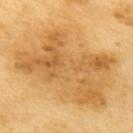Notes:
• follow-up — catalogued during a skin exam; not biopsied
• lighting — cross-polarized
• automated metrics — an area of roughly 80 mm², a shape eccentricity near 0.75, and a symmetry-axis asymmetry near 0.25; a mean CIELAB color near L≈63 a*≈19 b*≈46, a lesion–skin lightness drop of about 10, and a normalized border contrast of about 6.5; a border-irregularity rating of about 3/10 and a peripheral color-asymmetry measure near 2.5
• patient — male, aged approximately 60
• image — 15 mm crop, total-body photography
• location — the upper back
• lesion size — ≈12.5 mm